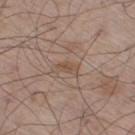biopsy_status: not biopsied; imaged during a skin examination
site: right thigh
image:
  source: total-body photography crop
  field_of_view_mm: 15
lighting: white-light
patient:
  sex: male
  age_approx: 60
lesion_size:
  long_diameter_mm_approx: 3.0